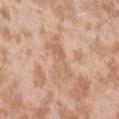biopsy_status: not biopsied; imaged during a skin examination
automated_metrics:
  nevus_likeness_0_100: 0
  lesion_detection_confidence_0_100: 80
site: left upper arm
image:
  source: total-body photography crop
  field_of_view_mm: 15
patient:
  sex: female
  age_approx: 25
lesion_size:
  long_diameter_mm_approx: 5.0